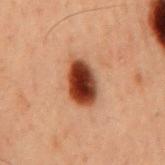biopsy status — catalogued during a skin exam; not biopsied
location — the mid back
tile lighting — cross-polarized
subject — male, aged approximately 60
image — 15 mm crop, total-body photography